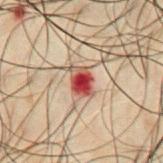Q: Was a biopsy performed?
A: no biopsy performed (imaged during a skin exam)
Q: Illumination type?
A: cross-polarized illumination
Q: Lesion location?
A: the abdomen
Q: Lesion size?
A: ~3 mm (longest diameter)
Q: What is the imaging modality?
A: ~15 mm tile from a whole-body skin photo
Q: Patient demographics?
A: male, about 50 years old